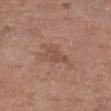The lesion was photographed on a routine skin check and not biopsied; there is no pathology result.
The subject is a male in their 60s.
The tile uses white-light illumination.
Cropped from a whole-body photographic skin survey; the tile spans about 15 mm.
On the right upper arm.